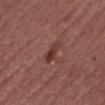Impression: Captured during whole-body skin photography for melanoma surveillance; the lesion was not biopsied. Context: The total-body-photography lesion software estimated a symmetry-axis asymmetry near 0.3. And it measured border irregularity of about 3.5 on a 0–10 scale, a within-lesion color-variation index near 0.5/10, and peripheral color asymmetry of about 0. A male subject approximately 55 years of age. Captured under white-light illumination. From the chest. The recorded lesion diameter is about 3.5 mm. This image is a 15 mm lesion crop taken from a total-body photograph.